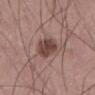Context: A male subject, aged 68 to 72. The lesion is on the left thigh. A close-up tile cropped from a whole-body skin photograph, about 15 mm across. The tile uses white-light illumination.